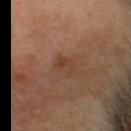Impression: The lesion was photographed on a routine skin check and not biopsied; there is no pathology result. Image and clinical context: The tile uses cross-polarized illumination. A male patient roughly 60 years of age. Measured at roughly 3 mm in maximum diameter. A roughly 15 mm field-of-view crop from a total-body skin photograph. On the head or neck.